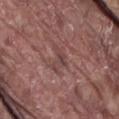The lesion was photographed on a routine skin check and not biopsied; there is no pathology result.
Approximately 3.5 mm at its widest.
The subject is a male aged 78–82.
A roughly 15 mm field-of-view crop from a total-body skin photograph.
On the lower back.
The lesion-visualizer software estimated an average lesion color of about L≈43 a*≈20 b*≈20 (CIELAB), roughly 7 lightness units darker than nearby skin, and a normalized border contrast of about 5.5. The analysis additionally found border irregularity of about 6.5 on a 0–10 scale, internal color variation of about 1.5 on a 0–10 scale, and a peripheral color-asymmetry measure near 0.5.